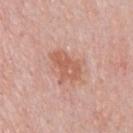The lesion was photographed on a routine skin check and not biopsied; there is no pathology result. A 15 mm crop from a total-body photograph taken for skin-cancer surveillance. Located on the chest. A male subject, about 60 years old.